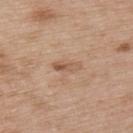<record>
<biopsy_status>not biopsied; imaged during a skin examination</biopsy_status>
<automated_metrics>
  <area_mm2_approx>3.5</area_mm2_approx>
  <eccentricity>0.95</eccentricity>
  <shape_asymmetry>0.2</shape_asymmetry>
  <border_irregularity_0_10>2.5</border_irregularity_0_10>
  <color_variation_0_10>1.0</color_variation_0_10>
  <peripheral_color_asymmetry>0.0</peripheral_color_asymmetry>
</automated_metrics>
<lighting>white-light</lighting>
<patient>
  <sex>male</sex>
  <age_approx>50</age_approx>
</patient>
<site>back</site>
<image>
  <source>total-body photography crop</source>
  <field_of_view_mm>15</field_of_view_mm>
</image>
<lesion_size>
  <long_diameter_mm_approx>3.5</long_diameter_mm_approx>
</lesion_size>
</record>